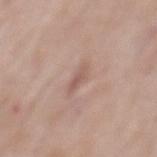follow-up=total-body-photography surveillance lesion; no biopsy
patient=male, aged around 55
image=~15 mm tile from a whole-body skin photo
location=the mid back